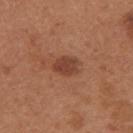workup: imaged on a skin check; not biopsied | lighting: white-light | diameter: ≈3 mm | image source: total-body-photography crop, ~15 mm field of view | patient: female, approximately 30 years of age | site: the arm.